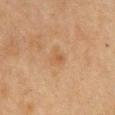workup = no biopsy performed (imaged during a skin exam)
tile lighting = cross-polarized illumination
patient = female, approximately 40 years of age
automated lesion analysis = an area of roughly 4 mm², a shape eccentricity near 0.55, and a symmetry-axis asymmetry near 0.35; a border-irregularity index near 3/10 and peripheral color asymmetry of about 1; a classifier nevus-likeness of about 0/100 and a lesion-detection confidence of about 100/100
size = about 2.5 mm
location = the chest
image = ~15 mm crop, total-body skin-cancer survey